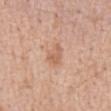Case summary:
• image source · total-body-photography crop, ~15 mm field of view
• subject · male, aged around 60
• location · the front of the torso
• tile lighting · white-light illumination
• lesion size · ≈2.5 mm
• automated lesion analysis · border irregularity of about 4.5 on a 0–10 scale, internal color variation of about 1.5 on a 0–10 scale, and peripheral color asymmetry of about 0.5; lesion-presence confidence of about 100/100